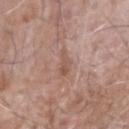Impression:
Imaged during a routine full-body skin examination; the lesion was not biopsied and no histopathology is available.
Background:
A male patient aged around 75. On the right forearm. A 15 mm crop from a total-body photograph taken for skin-cancer surveillance. Imaged with white-light lighting. The recorded lesion diameter is about 3.5 mm.